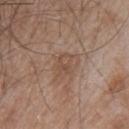Part of a total-body skin-imaging series; this lesion was reviewed on a skin check and was not flagged for biopsy.
The tile uses white-light illumination.
The subject is a male aged around 55.
Longest diameter approximately 2.5 mm.
A 15 mm close-up extracted from a 3D total-body photography capture.
Located on the back.